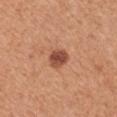notes: no biopsy performed (imaged during a skin exam)
subject: female, in their mid-40s
lighting: white-light
anatomic site: the left upper arm
image: 15 mm crop, total-body photography
image-analysis metrics: a footprint of about 5 mm² and a shape eccentricity near 0.55; a border-irregularity rating of about 1.5/10 and a peripheral color-asymmetry measure near 1.5
diameter: ~2.5 mm (longest diameter)